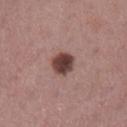| feature | finding |
|---|---|
| follow-up | imaged on a skin check; not biopsied |
| location | the left lower leg |
| illumination | white-light |
| imaging modality | 15 mm crop, total-body photography |
| patient | female, approximately 60 years of age |
| image-analysis metrics | a mean CIELAB color near L≈41 a*≈20 b*≈20, a lesion–skin lightness drop of about 17, and a normalized lesion–skin contrast near 12.5; a border-irregularity index near 1.5/10, a within-lesion color-variation index near 3.5/10, and radial color variation of about 1 |
| diameter | about 3 mm |